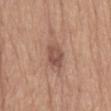Imaged during a routine full-body skin examination; the lesion was not biopsied and no histopathology is available. Located on the abdomen. Imaged with white-light lighting. A roughly 15 mm field-of-view crop from a total-body skin photograph. An algorithmic analysis of the crop reported an area of roughly 6.5 mm², an outline eccentricity of about 0.8 (0 = round, 1 = elongated), and a symmetry-axis asymmetry near 0.25. The analysis additionally found a mean CIELAB color near L≈51 a*≈21 b*≈26, about 10 CIELAB-L* units darker than the surrounding skin, and a lesion-to-skin contrast of about 7.5 (normalized; higher = more distinct). And it measured a border-irregularity index near 2.5/10. The subject is a male approximately 70 years of age. The recorded lesion diameter is about 3.5 mm.